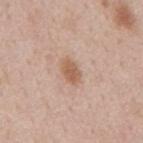Part of a total-body skin-imaging series; this lesion was reviewed on a skin check and was not flagged for biopsy.
Cropped from a whole-body photographic skin survey; the tile spans about 15 mm.
A male subject, about 50 years old.
Longest diameter approximately 3 mm.
From the mid back.
Captured under white-light illumination.